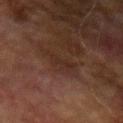Imaged during a routine full-body skin examination; the lesion was not biopsied and no histopathology is available.
A lesion tile, about 15 mm wide, cut from a 3D total-body photograph.
The lesion is on the left forearm.
Approximately 4 mm at its widest.
A male patient, aged 73–77.
Captured under cross-polarized illumination.